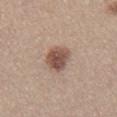Cropped from a whole-body photographic skin survey; the tile spans about 15 mm.
The subject is a male about 55 years old.
From the chest.